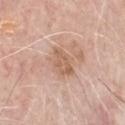  biopsy_status: not biopsied; imaged during a skin examination
  automated_metrics:
    area_mm2_approx: 8.0
    shape_asymmetry: 0.25
    vs_skin_darker_L: 8.0
    border_irregularity_0_10: 2.5
    color_variation_0_10: 3.0
    peripheral_color_asymmetry: 1.0
    nevus_likeness_0_100: 0
    lesion_detection_confidence_0_100: 100
  image:
    source: total-body photography crop
    field_of_view_mm: 15
  site: chest
  lesion_size:
    long_diameter_mm_approx: 4.0
  patient:
    sex: male
    age_approx: 75